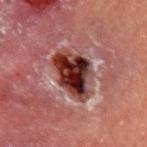No biopsy was performed on this lesion — it was imaged during a full skin examination and was not determined to be concerning. A 15 mm close-up extracted from a 3D total-body photography capture. The recorded lesion diameter is about 5.5 mm. The lesion is located on the left upper arm. The subject is a male aged 53 to 57. The tile uses cross-polarized illumination.